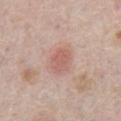Clinical impression:
The lesion was tiled from a total-body skin photograph and was not biopsied.
Background:
Imaged with white-light lighting. The subject is a male aged 73–77. Automated tile analysis of the lesion measured an area of roughly 6 mm², an outline eccentricity of about 0.7 (0 = round, 1 = elongated), and a shape-asymmetry score of about 0.2 (0 = symmetric). And it measured a mean CIELAB color near L≈59 a*≈23 b*≈25, about 8 CIELAB-L* units darker than the surrounding skin, and a normalized lesion–skin contrast near 5.5. And it measured peripheral color asymmetry of about 1. It also reported a classifier nevus-likeness of about 85/100. A 15 mm close-up extracted from a 3D total-body photography capture. From the abdomen.